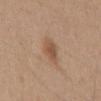Findings:
- workup — no biopsy performed (imaged during a skin exam)
- image — ~15 mm tile from a whole-body skin photo
- body site — the chest
- lesion size — ~3.5 mm (longest diameter)
- automated lesion analysis — a border-irregularity index near 2/10, internal color variation of about 3 on a 0–10 scale, and peripheral color asymmetry of about 1
- subject — male, approximately 30 years of age
- illumination — white-light illumination